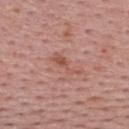This lesion was catalogued during total-body skin photography and was not selected for biopsy.
Cropped from a whole-body photographic skin survey; the tile spans about 15 mm.
A female patient, aged around 55.
Automated image analysis of the tile measured a mean CIELAB color near L≈54 a*≈25 b*≈27 and a lesion–skin lightness drop of about 8. The software also gave a classifier nevus-likeness of about 0/100 and a detector confidence of about 100 out of 100 that the crop contains a lesion.
Measured at roughly 4 mm in maximum diameter.
Imaged with white-light lighting.
The lesion is on the upper back.